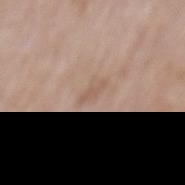Clinical summary: Longest diameter approximately 2.5 mm. The lesion is on the mid back. The subject is a female about 75 years old. A lesion tile, about 15 mm wide, cut from a 3D total-body photograph. This is a white-light tile.